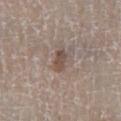Impression:
Part of a total-body skin-imaging series; this lesion was reviewed on a skin check and was not flagged for biopsy.
Acquisition and patient details:
The lesion is on the right lower leg. A male patient, in their 60s. About 3 mm across. A roughly 15 mm field-of-view crop from a total-body skin photograph. This is a white-light tile.